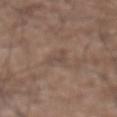<lesion>
<biopsy_status>not biopsied; imaged during a skin examination</biopsy_status>
<patient>
  <sex>male</sex>
  <age_approx>75</age_approx>
</patient>
<image>
  <source>total-body photography crop</source>
  <field_of_view_mm>15</field_of_view_mm>
</image>
<lesion_size>
  <long_diameter_mm_approx>3.0</long_diameter_mm_approx>
</lesion_size>
<site>front of the torso</site>
<lighting>white-light</lighting>
</lesion>